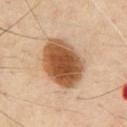| field | value |
|---|---|
| workup | imaged on a skin check; not biopsied |
| patient | male, about 70 years old |
| illumination | cross-polarized |
| lesion size | about 6 mm |
| location | the chest |
| acquisition | 15 mm crop, total-body photography |